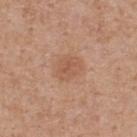| feature | finding |
|---|---|
| biopsy status | no biopsy performed (imaged during a skin exam) |